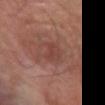This lesion was catalogued during total-body skin photography and was not selected for biopsy. An algorithmic analysis of the crop reported an area of roughly 5 mm², a shape eccentricity near 0.9, and a symmetry-axis asymmetry near 0.35. And it measured roughly 6 lightness units darker than nearby skin. And it measured a border-irregularity rating of about 4/10, internal color variation of about 1.5 on a 0–10 scale, and radial color variation of about 0.5. The analysis additionally found a nevus-likeness score of about 5/100 and a detector confidence of about 95 out of 100 that the crop contains a lesion. Located on the left forearm. Cropped from a whole-body photographic skin survey; the tile spans about 15 mm.